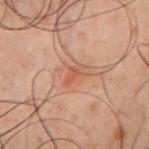Imaged during a routine full-body skin examination; the lesion was not biopsied and no histopathology is available.
An algorithmic analysis of the crop reported a lesion area of about 3.5 mm² and an outline eccentricity of about 0.85 (0 = round, 1 = elongated). And it measured an average lesion color of about L≈42 a*≈20 b*≈26 (CIELAB) and roughly 6 lightness units darker than nearby skin. The analysis additionally found a border-irregularity rating of about 3/10, a color-variation rating of about 2/10, and a peripheral color-asymmetry measure near 0.5. And it measured a nevus-likeness score of about 5/100 and a detector confidence of about 100 out of 100 that the crop contains a lesion.
A male subject, aged 58 to 62.
Captured under cross-polarized illumination.
The lesion's longest dimension is about 3 mm.
The lesion is on the chest.
This image is a 15 mm lesion crop taken from a total-body photograph.